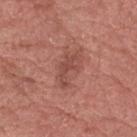<lesion>
<biopsy_status>not biopsied; imaged during a skin examination</biopsy_status>
<automated_metrics>
  <cielab_L>48</cielab_L>
  <cielab_a>25</cielab_a>
  <cielab_b>26</cielab_b>
  <vs_skin_contrast_norm>6.0</vs_skin_contrast_norm>
  <border_irregularity_0_10>6.0</border_irregularity_0_10>
  <color_variation_0_10>2.0</color_variation_0_10>
  <peripheral_color_asymmetry>0.5</peripheral_color_asymmetry>
</automated_metrics>
<lesion_size>
  <long_diameter_mm_approx>4.0</long_diameter_mm_approx>
</lesion_size>
<image>
  <source>total-body photography crop</source>
  <field_of_view_mm>15</field_of_view_mm>
</image>
<site>head or neck</site>
<patient>
  <sex>male</sex>
  <age_approx>75</age_approx>
</patient>
<lighting>white-light</lighting>
</lesion>